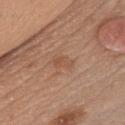The patient is a male about 45 years old. Imaged with white-light lighting. Cropped from a total-body skin-imaging series; the visible field is about 15 mm. Approximately 2.5 mm at its widest. The lesion is located on the chest.